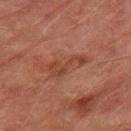<lesion>
  <biopsy_status>not biopsied; imaged during a skin examination</biopsy_status>
  <site>right thigh</site>
  <image>
    <source>total-body photography crop</source>
    <field_of_view_mm>15</field_of_view_mm>
  </image>
  <patient>
    <sex>male</sex>
    <age_approx>75</age_approx>
  </patient>
  <lesion_size>
    <long_diameter_mm_approx>4.5</long_diameter_mm_approx>
  </lesion_size>
  <lighting>cross-polarized</lighting>
</lesion>